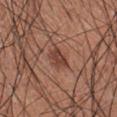– follow-up — imaged on a skin check; not biopsied
– patient — male, about 35 years old
– lighting — white-light illumination
– automated metrics — an area of roughly 4.5 mm², an outline eccentricity of about 0.85 (0 = round, 1 = elongated), and two-axis asymmetry of about 0.3; a mean CIELAB color near L≈42 a*≈23 b*≈26, about 10 CIELAB-L* units darker than the surrounding skin, and a normalized border contrast of about 8; border irregularity of about 3.5 on a 0–10 scale, a color-variation rating of about 2/10, and a peripheral color-asymmetry measure near 1; a nevus-likeness score of about 75/100 and a lesion-detection confidence of about 100/100
– imaging modality — total-body-photography crop, ~15 mm field of view
– location — the left upper arm